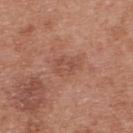Q: Was a biopsy performed?
A: imaged on a skin check; not biopsied